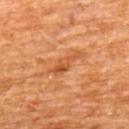Case summary:
* workup — catalogued during a skin exam; not biopsied
* site — the back
* patient — male, in their mid-60s
* image source — ~15 mm tile from a whole-body skin photo